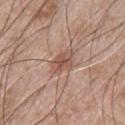workup = imaged on a skin check; not biopsied | subject = male, aged approximately 50 | acquisition = ~15 mm crop, total-body skin-cancer survey | size = about 3.5 mm | anatomic site = the chest | lighting = white-light.